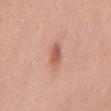Context:
A female subject, approximately 35 years of age. The tile uses white-light illumination. A close-up tile cropped from a whole-body skin photograph, about 15 mm across. On the abdomen. The recorded lesion diameter is about 2.5 mm. An algorithmic analysis of the crop reported an area of roughly 4 mm². And it measured a mean CIELAB color near L≈58 a*≈26 b*≈30. The analysis additionally found border irregularity of about 1.5 on a 0–10 scale and a within-lesion color-variation index near 2.5/10. The analysis additionally found a nevus-likeness score of about 80/100 and a detector confidence of about 100 out of 100 that the crop contains a lesion.